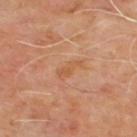<case>
<biopsy_status>not biopsied; imaged during a skin examination</biopsy_status>
<lesion_size>
  <long_diameter_mm_approx>3.5</long_diameter_mm_approx>
</lesion_size>
<site>upper back</site>
<image>
  <source>total-body photography crop</source>
  <field_of_view_mm>15</field_of_view_mm>
</image>
<automated_metrics>
  <vs_skin_darker_L>6.0</vs_skin_darker_L>
</automated_metrics>
<patient>
  <sex>male</sex>
  <age_approx>65</age_approx>
</patient>
</case>